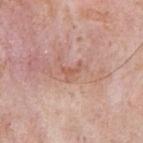| feature | finding |
|---|---|
| follow-up | imaged on a skin check; not biopsied |
| subject | male, aged around 70 |
| body site | the right upper arm |
| diameter | ≈2.5 mm |
| image source | 15 mm crop, total-body photography |
| tile lighting | white-light |